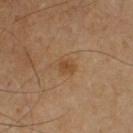illumination: cross-polarized; patient: male, aged 58–62; imaging modality: ~15 mm tile from a whole-body skin photo; diameter: ~2 mm (longest diameter); site: the right upper arm.